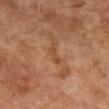Q: Was a biopsy performed?
A: total-body-photography surveillance lesion; no biopsy
Q: What lighting was used for the tile?
A: cross-polarized illumination
Q: What is the imaging modality?
A: ~15 mm crop, total-body skin-cancer survey
Q: What is the anatomic site?
A: the leg
Q: Patient demographics?
A: male, roughly 65 years of age
Q: Lesion size?
A: ~3.5 mm (longest diameter)